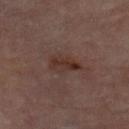biopsy status — catalogued during a skin exam; not biopsied
diameter — ≈3.5 mm
lighting — cross-polarized
automated metrics — an area of roughly 5.5 mm², an outline eccentricity of about 0.85 (0 = round, 1 = elongated), and a shape-asymmetry score of about 0.3 (0 = symmetric); a mean CIELAB color near L≈28 a*≈16 b*≈20, roughly 7 lightness units darker than nearby skin, and a normalized lesion–skin contrast near 7.5; a border-irregularity rating of about 3.5/10, a color-variation rating of about 3.5/10, and peripheral color asymmetry of about 1; a classifier nevus-likeness of about 30/100 and lesion-presence confidence of about 100/100
subject — female, about 80 years old
image — total-body-photography crop, ~15 mm field of view
location — the left leg